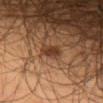biopsy_status: not biopsied; imaged during a skin examination
image:
  source: total-body photography crop
  field_of_view_mm: 15
lighting: cross-polarized
patient:
  sex: male
  age_approx: 45
site: right upper arm
automated_metrics:
  area_mm2_approx: 4.5
  eccentricity: 0.85
  shape_asymmetry: 0.25
  cielab_L: 36
  cielab_a: 20
  cielab_b: 30
  vs_skin_darker_L: 10.0
  vs_skin_contrast_norm: 8.5
  lesion_detection_confidence_0_100: 100
lesion_size:
  long_diameter_mm_approx: 3.0